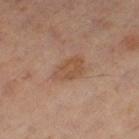Captured during whole-body skin photography for melanoma surveillance; the lesion was not biopsied. The lesion is on the leg. A lesion tile, about 15 mm wide, cut from a 3D total-body photograph. A male patient, aged 58–62.